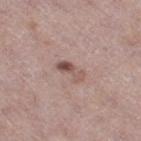Recorded during total-body skin imaging; not selected for excision or biopsy. Located on the leg. A lesion tile, about 15 mm wide, cut from a 3D total-body photograph. A female patient, in their 60s.